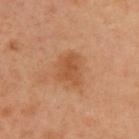Q: Is there a histopathology result?
A: imaged on a skin check; not biopsied
Q: Where on the body is the lesion?
A: the upper back
Q: What are the patient's age and sex?
A: male, aged around 40
Q: What did automated image analysis measure?
A: an outline eccentricity of about 0.65 (0 = round, 1 = elongated); a lesion color around L≈51 a*≈24 b*≈37 in CIELAB and a normalized border contrast of about 6.5; border irregularity of about 2.5 on a 0–10 scale, internal color variation of about 2 on a 0–10 scale, and radial color variation of about 1; an automated nevus-likeness rating near 15 out of 100
Q: Illumination type?
A: cross-polarized illumination
Q: How was this image acquired?
A: ~15 mm tile from a whole-body skin photo
Q: What is the lesion's diameter?
A: about 3.5 mm